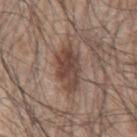This lesion was catalogued during total-body skin photography and was not selected for biopsy. A region of skin cropped from a whole-body photographic capture, roughly 15 mm wide. About 5.5 mm across. The tile uses white-light illumination. The lesion is on the arm. The subject is a male aged around 70.